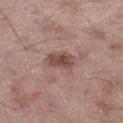  biopsy_status: not biopsied; imaged during a skin examination
  site: leg
  lighting: white-light
  lesion_size:
    long_diameter_mm_approx: 3.5
  image:
    source: total-body photography crop
    field_of_view_mm: 15
  patient:
    sex: male
    age_approx: 50